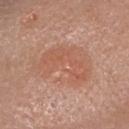follow-up: no biopsy performed (imaged during a skin exam) | image source: ~15 mm crop, total-body skin-cancer survey | automated lesion analysis: a footprint of about 22 mm², an outline eccentricity of about 0.8 (0 = round, 1 = elongated), and a shape-asymmetry score of about 0.4 (0 = symmetric); lesion-presence confidence of about 100/100 | body site: the head or neck | tile lighting: white-light | patient: female, in their 40s.